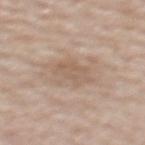Notes:
– biopsy status · no biopsy performed (imaged during a skin exam)
– subject · female, approximately 60 years of age
– lesion diameter · ≈4 mm
– site · the upper back
– imaging modality · total-body-photography crop, ~15 mm field of view
– lighting · white-light